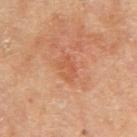notes=total-body-photography surveillance lesion; no biopsy
imaging modality=15 mm crop, total-body photography
lesion diameter=~2.5 mm (longest diameter)
site=the right upper arm
patient=female, aged around 65